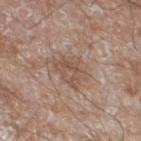Imaged during a routine full-body skin examination; the lesion was not biopsied and no histopathology is available. The subject is a male aged approximately 60. Captured under white-light illumination. A close-up tile cropped from a whole-body skin photograph, about 15 mm across. Automated tile analysis of the lesion measured a border-irregularity rating of about 4/10, a within-lesion color-variation index near 4/10, and a peripheral color-asymmetry measure near 1.5. It also reported a nevus-likeness score of about 0/100. On the leg.